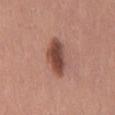Impression:
This lesion was catalogued during total-body skin photography and was not selected for biopsy.
Context:
The recorded lesion diameter is about 5 mm. The patient is a male about 30 years old. Captured under white-light illumination. A lesion tile, about 15 mm wide, cut from a 3D total-body photograph. The lesion-visualizer software estimated a lesion area of about 10 mm² and a shape-asymmetry score of about 0.2 (0 = symmetric). The analysis additionally found a mean CIELAB color near L≈47 a*≈23 b*≈26 and about 14 CIELAB-L* units darker than the surrounding skin. It also reported a nevus-likeness score of about 95/100 and lesion-presence confidence of about 100/100. On the mid back.